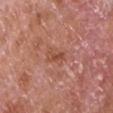Q: Was a biopsy performed?
A: no biopsy performed (imaged during a skin exam)
Q: Who is the patient?
A: male, approximately 65 years of age
Q: Illumination type?
A: white-light
Q: How was this image acquired?
A: ~15 mm crop, total-body skin-cancer survey
Q: Automated lesion metrics?
A: a border-irregularity index near 4/10 and a color-variation rating of about 0/10; an automated nevus-likeness rating near 0 out of 100 and lesion-presence confidence of about 100/100
Q: How large is the lesion?
A: ~3 mm (longest diameter)
Q: What is the anatomic site?
A: the chest